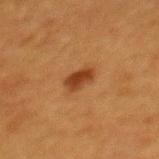Notes:
• notes — catalogued during a skin exam; not biopsied
• anatomic site — the upper back
• lighting — cross-polarized
• lesion size — ~3 mm (longest diameter)
• acquisition — ~15 mm tile from a whole-body skin photo
• patient — female, roughly 40 years of age
• TBP lesion metrics — a lesion area of about 5 mm² and a shape-asymmetry score of about 0.2 (0 = symmetric); an average lesion color of about L≈34 a*≈22 b*≈33 (CIELAB), roughly 10 lightness units darker than nearby skin, and a lesion-to-skin contrast of about 9 (normalized; higher = more distinct); a within-lesion color-variation index near 3/10 and a peripheral color-asymmetry measure near 1; a nevus-likeness score of about 100/100 and a lesion-detection confidence of about 100/100